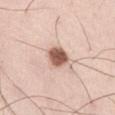follow-up = catalogued during a skin exam; not biopsied
acquisition = ~15 mm tile from a whole-body skin photo
site = the leg
illumination = white-light illumination
patient = male, aged 33–37